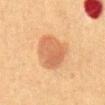notes = catalogued during a skin exam; not biopsied
site = the abdomen
subject = male, aged around 50
lesion size = about 5 mm
image source = total-body-photography crop, ~15 mm field of view
tile lighting = cross-polarized